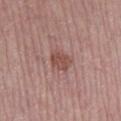<record>
  <biopsy_status>not biopsied; imaged during a skin examination</biopsy_status>
  <lesion_size>
    <long_diameter_mm_approx>3.0</long_diameter_mm_approx>
  </lesion_size>
  <image>
    <source>total-body photography crop</source>
    <field_of_view_mm>15</field_of_view_mm>
  </image>
  <patient>
    <sex>male</sex>
    <age_approx>75</age_approx>
  </patient>
  <automated_metrics>
    <area_mm2_approx>5.0</area_mm2_approx>
    <eccentricity>0.65</eccentricity>
    <shape_asymmetry>0.15</shape_asymmetry>
    <vs_skin_darker_L>9.0</vs_skin_darker_L>
    <vs_skin_contrast_norm>7.0</vs_skin_contrast_norm>
  </automated_metrics>
  <lighting>white-light</lighting>
  <site>right lower leg</site>
</record>